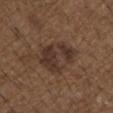Assessment: The lesion was tiled from a total-body skin photograph and was not biopsied. Image and clinical context: Located on the right upper arm. A male patient, aged approximately 50. A lesion tile, about 15 mm wide, cut from a 3D total-body photograph.